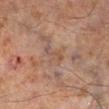Assessment:
This lesion was catalogued during total-body skin photography and was not selected for biopsy.
Acquisition and patient details:
Approximately 3.5 mm at its widest. A roughly 15 mm field-of-view crop from a total-body skin photograph. Automated image analysis of the tile measured an automated nevus-likeness rating near 0 out of 100. The lesion is on the leg. Imaged with cross-polarized lighting. A male patient, aged 63 to 67.